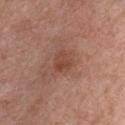Clinical summary: From the chest. The patient is a male roughly 65 years of age. Cropped from a total-body skin-imaging series; the visible field is about 15 mm. An algorithmic analysis of the crop reported a border-irregularity rating of about 3/10, a within-lesion color-variation index near 3/10, and radial color variation of about 1. The analysis additionally found lesion-presence confidence of about 100/100. About 2.5 mm across.